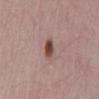follow-up: imaged on a skin check; not biopsied | subject: male, aged around 70 | anatomic site: the mid back | tile lighting: white-light | lesion diameter: ≈3 mm | image source: 15 mm crop, total-body photography.